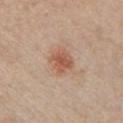- workup · catalogued during a skin exam; not biopsied
- size · ≈3.5 mm
- anatomic site · the chest
- subject · female, approximately 45 years of age
- TBP lesion metrics · a footprint of about 7.5 mm² and two-axis asymmetry of about 0.2; a mean CIELAB color near L≈56 a*≈22 b*≈31 and about 10 CIELAB-L* units darker than the surrounding skin; a border-irregularity rating of about 2/10, a color-variation rating of about 4/10, and radial color variation of about 1; a detector confidence of about 100 out of 100 that the crop contains a lesion
- image · 15 mm crop, total-body photography